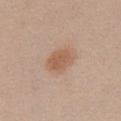The lesion was tiled from a total-body skin photograph and was not biopsied. Captured under white-light illumination. A female subject aged 38 to 42. Cropped from a whole-body photographic skin survey; the tile spans about 15 mm. Automated image analysis of the tile measured a lesion area of about 7.5 mm² and an eccentricity of roughly 0.65. It also reported a mean CIELAB color near L≈57 a*≈20 b*≈30, about 9 CIELAB-L* units darker than the surrounding skin, and a lesion-to-skin contrast of about 7 (normalized; higher = more distinct). It also reported border irregularity of about 2 on a 0–10 scale and peripheral color asymmetry of about 1. The lesion is located on the chest. The lesion's longest dimension is about 3.5 mm.